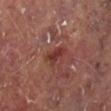| key | value |
|---|---|
| follow-up | no biopsy performed (imaged during a skin exam) |
| tile lighting | cross-polarized illumination |
| patient | male, aged approximately 65 |
| location | the leg |
| image source | ~15 mm crop, total-body skin-cancer survey |